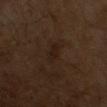Imaged during a routine full-body skin examination; the lesion was not biopsied and no histopathology is available.
A close-up tile cropped from a whole-body skin photograph, about 15 mm across.
A male subject, in their mid- to late 60s.